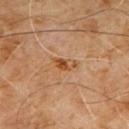Impression:
The lesion was tiled from a total-body skin photograph and was not biopsied.
Acquisition and patient details:
The patient is a male aged around 60. This image is a 15 mm lesion crop taken from a total-body photograph. From the front of the torso.